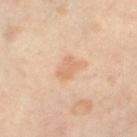No biopsy was performed on this lesion — it was imaged during a full skin examination and was not determined to be concerning.
Imaged with cross-polarized lighting.
The lesion is on the leg.
A lesion tile, about 15 mm wide, cut from a 3D total-body photograph.
A female patient approximately 50 years of age.
Approximately 2.5 mm at its widest.